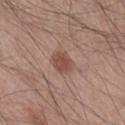follow-up: catalogued during a skin exam; not biopsied
body site: the right lower leg
subject: male, aged around 45
image: 15 mm crop, total-body photography
lesion size: about 3 mm
automated metrics: an eccentricity of roughly 0.7 and a symmetry-axis asymmetry near 0.15; a mean CIELAB color near L≈49 a*≈20 b*≈26, about 9 CIELAB-L* units darker than the surrounding skin, and a normalized lesion–skin contrast near 7; border irregularity of about 1.5 on a 0–10 scale, internal color variation of about 2 on a 0–10 scale, and a peripheral color-asymmetry measure near 0.5